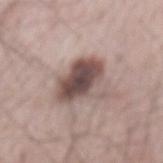Image and clinical context: The subject is a male aged 63–67. A 15 mm close-up extracted from a 3D total-body photography capture. On the back. The recorded lesion diameter is about 5 mm.